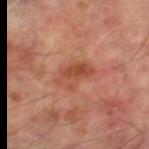{"biopsy_status": "not biopsied; imaged during a skin examination", "patient": {"sex": "male", "age_approx": 65}, "lesion_size": {"long_diameter_mm_approx": 4.0}, "automated_metrics": {"area_mm2_approx": 8.5, "eccentricity": 0.7, "border_irregularity_0_10": 3.5, "color_variation_0_10": 5.0, "peripheral_color_asymmetry": 2.0, "nevus_likeness_0_100": 5, "lesion_detection_confidence_0_100": 100}, "site": "left lower leg", "lighting": "cross-polarized", "image": {"source": "total-body photography crop", "field_of_view_mm": 15}}